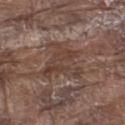Recorded during total-body skin imaging; not selected for excision or biopsy.
A male subject approximately 80 years of age.
A roughly 15 mm field-of-view crop from a total-body skin photograph.
An algorithmic analysis of the crop reported an average lesion color of about L≈40 a*≈17 b*≈23 (CIELAB), a lesion–skin lightness drop of about 8, and a normalized lesion–skin contrast near 6.5. And it measured a border-irregularity index near 7.5/10 and a within-lesion color-variation index near 3/10. And it measured an automated nevus-likeness rating near 0 out of 100 and a detector confidence of about 50 out of 100 that the crop contains a lesion.
The lesion's longest dimension is about 5 mm.
On the left thigh.
The tile uses white-light illumination.